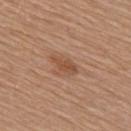Imaged during a routine full-body skin examination; the lesion was not biopsied and no histopathology is available. Cropped from a total-body skin-imaging series; the visible field is about 15 mm. The lesion is located on the back. Longest diameter approximately 3.5 mm. The tile uses white-light illumination. A female subject in their mid- to late 50s.